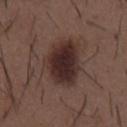  biopsy_status: not biopsied; imaged during a skin examination
  patient:
    sex: male
    age_approx: 50
  lighting: white-light
  lesion_size:
    long_diameter_mm_approx: 6.0
  site: mid back
  image:
    source: total-body photography crop
    field_of_view_mm: 15